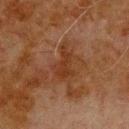follow-up = no biopsy performed (imaged during a skin exam).